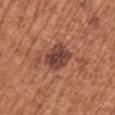Captured during whole-body skin photography for melanoma surveillance; the lesion was not biopsied.
This is a white-light tile.
A female patient, in their mid-70s.
A 15 mm close-up extracted from a 3D total-body photography capture.
The lesion's longest dimension is about 4 mm.
Located on the left upper arm.